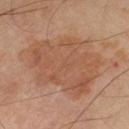<case>
  <biopsy_status>not biopsied; imaged during a skin examination</biopsy_status>
  <lighting>cross-polarized</lighting>
  <lesion_size>
    <long_diameter_mm_approx>10.0</long_diameter_mm_approx>
  </lesion_size>
  <image>
    <source>total-body photography crop</source>
    <field_of_view_mm>15</field_of_view_mm>
  </image>
  <patient>
    <sex>male</sex>
    <age_approx>65</age_approx>
  </patient>
  <site>leg</site>
</case>